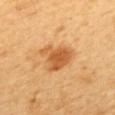Imaged during a routine full-body skin examination; the lesion was not biopsied and no histopathology is available.
The subject is a female in their mid-50s.
The lesion is located on the upper back.
Captured under cross-polarized illumination.
A lesion tile, about 15 mm wide, cut from a 3D total-body photograph.
Approximately 4.5 mm at its widest.
Automated image analysis of the tile measured an area of roughly 10 mm², a shape eccentricity near 0.7, and two-axis asymmetry of about 0.45. It also reported a mean CIELAB color near L≈59 a*≈26 b*≈46 and about 13 CIELAB-L* units darker than the surrounding skin. And it measured border irregularity of about 5 on a 0–10 scale, a color-variation rating of about 3/10, and radial color variation of about 1.